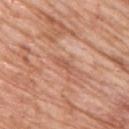| feature | finding |
|---|---|
| automated lesion analysis | a nevus-likeness score of about 0/100 and a detector confidence of about 50 out of 100 that the crop contains a lesion |
| location | the upper back |
| image | ~15 mm crop, total-body skin-cancer survey |
| subject | female, in their mid- to late 60s |